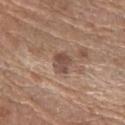Notes:
- workup — total-body-photography surveillance lesion; no biopsy
- image source — ~15 mm crop, total-body skin-cancer survey
- anatomic site — the right forearm
- subject — female, approximately 60 years of age